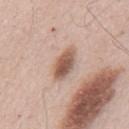Assessment:
The lesion was tiled from a total-body skin photograph and was not biopsied.
Background:
Longest diameter approximately 4.5 mm. A male patient, approximately 55 years of age. This image is a 15 mm lesion crop taken from a total-body photograph. Imaged with white-light lighting. From the mid back.